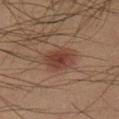Q: Is there a histopathology result?
A: imaged on a skin check; not biopsied
Q: What is the imaging modality?
A: 15 mm crop, total-body photography
Q: What are the patient's age and sex?
A: male, aged around 35
Q: What is the lesion's diameter?
A: about 3.5 mm
Q: Lesion location?
A: the right lower leg